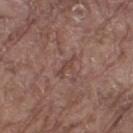No biopsy was performed on this lesion — it was imaged during a full skin examination and was not determined to be concerning.
The lesion is located on the right thigh.
A roughly 15 mm field-of-view crop from a total-body skin photograph.
The lesion-visualizer software estimated an eccentricity of roughly 0.95 and a symmetry-axis asymmetry near 0.4. It also reported a mean CIELAB color near L≈43 a*≈19 b*≈23, a lesion–skin lightness drop of about 7, and a normalized lesion–skin contrast near 5.5. And it measured a classifier nevus-likeness of about 0/100 and a detector confidence of about 75 out of 100 that the crop contains a lesion.
The patient is a male in their 80s.
About 3 mm across.
Captured under white-light illumination.